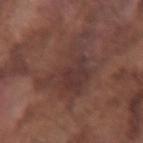A 15 mm crop from a total-body photograph taken for skin-cancer surveillance. The patient is a male aged 73–77. The lesion is located on the right forearm.